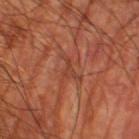Imaged during a routine full-body skin examination; the lesion was not biopsied and no histopathology is available. Approximately 3 mm at its widest. A male subject aged 58 to 62. A region of skin cropped from a whole-body photographic capture, roughly 15 mm wide. Automated tile analysis of the lesion measured an outline eccentricity of about 0.85 (0 = round, 1 = elongated) and a symmetry-axis asymmetry near 0.7. The analysis additionally found a classifier nevus-likeness of about 0/100 and a detector confidence of about 75 out of 100 that the crop contains a lesion. The lesion is on the leg.